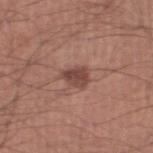No biopsy was performed on this lesion — it was imaged during a full skin examination and was not determined to be concerning. The total-body-photography lesion software estimated an area of roughly 5.5 mm² and an outline eccentricity of about 0.65 (0 = round, 1 = elongated). The software also gave a mean CIELAB color near L≈45 a*≈21 b*≈23, about 11 CIELAB-L* units darker than the surrounding skin, and a normalized border contrast of about 8. And it measured a border-irregularity rating of about 3/10 and radial color variation of about 1. And it measured a classifier nevus-likeness of about 80/100 and a detector confidence of about 100 out of 100 that the crop contains a lesion. A roughly 15 mm field-of-view crop from a total-body skin photograph. A male patient about 45 years old. The tile uses white-light illumination. Measured at roughly 3 mm in maximum diameter. Located on the right thigh.